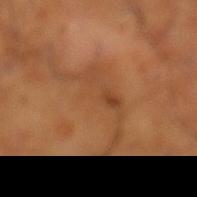The lesion was tiled from a total-body skin photograph and was not biopsied. On the left lower leg. The patient is a male about 65 years old. A lesion tile, about 15 mm wide, cut from a 3D total-body photograph. This is a cross-polarized tile. Measured at roughly 9 mm in maximum diameter. Automated image analysis of the tile measured an average lesion color of about L≈46 a*≈22 b*≈35 (CIELAB) and about 6 CIELAB-L* units darker than the surrounding skin.